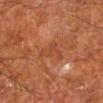{"biopsy_status": "not biopsied; imaged during a skin examination", "image": {"source": "total-body photography crop", "field_of_view_mm": 15}, "lesion_size": {"long_diameter_mm_approx": 3.5}, "site": "leg", "patient": {"sex": "male", "age_approx": 65}, "automated_metrics": {"eccentricity": 0.85, "shape_asymmetry": 0.6, "border_irregularity_0_10": 6.5, "peripheral_color_asymmetry": 0.5}, "lighting": "cross-polarized"}